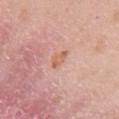Captured during whole-body skin photography for melanoma surveillance; the lesion was not biopsied.
The lesion-visualizer software estimated a mean CIELAB color near L≈62 a*≈24 b*≈31 and a lesion–skin lightness drop of about 8. The software also gave a color-variation rating of about 1/10 and a peripheral color-asymmetry measure near 0.5. And it measured a nevus-likeness score of about 0/100 and a detector confidence of about 100 out of 100 that the crop contains a lesion.
The subject is a female in their 50s.
The tile uses white-light illumination.
Longest diameter approximately 3 mm.
A 15 mm close-up tile from a total-body photography series done for melanoma screening.
From the right upper arm.